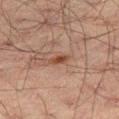Clinical impression:
The lesion was photographed on a routine skin check and not biopsied; there is no pathology result.
Context:
The lesion's longest dimension is about 3 mm. The tile uses cross-polarized illumination. A roughly 15 mm field-of-view crop from a total-body skin photograph. From the right thigh. The lesion-visualizer software estimated a color-variation rating of about 1/10 and a peripheral color-asymmetry measure near 0. A male patient aged 48–52.